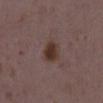The lesion was tiled from a total-body skin photograph and was not biopsied.
A female patient roughly 35 years of age.
The tile uses white-light illumination.
An algorithmic analysis of the crop reported a lesion area of about 6 mm², an outline eccentricity of about 0.6 (0 = round, 1 = elongated), and a symmetry-axis asymmetry near 0.25. The analysis additionally found an average lesion color of about L≈33 a*≈17 b*≈21 (CIELAB), a lesion–skin lightness drop of about 10, and a normalized lesion–skin contrast near 10. It also reported border irregularity of about 2 on a 0–10 scale, a color-variation rating of about 2.5/10, and peripheral color asymmetry of about 0.5. And it measured an automated nevus-likeness rating near 95 out of 100 and a lesion-detection confidence of about 100/100.
On the right lower leg.
A 15 mm close-up extracted from a 3D total-body photography capture.
About 3 mm across.